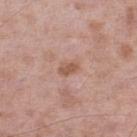follow-up — no biopsy performed (imaged during a skin exam)
lesion diameter — ~2.5 mm (longest diameter)
patient — male, in their mid-50s
site — the left lower leg
acquisition — 15 mm crop, total-body photography
tile lighting — white-light